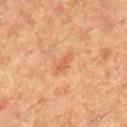Q: Was this lesion biopsied?
A: catalogued during a skin exam; not biopsied
Q: What are the patient's age and sex?
A: female, roughly 50 years of age
Q: What is the anatomic site?
A: the right lower leg
Q: How was this image acquired?
A: total-body-photography crop, ~15 mm field of view